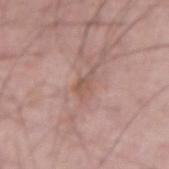Q: Is there a histopathology result?
A: imaged on a skin check; not biopsied
Q: Lesion location?
A: the right forearm
Q: How was this image acquired?
A: ~15 mm crop, total-body skin-cancer survey
Q: Patient demographics?
A: male, aged around 50